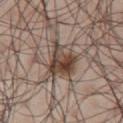Impression:
No biopsy was performed on this lesion — it was imaged during a full skin examination and was not determined to be concerning.
Context:
The lesion's longest dimension is about 5 mm. This is a white-light tile. A male subject, in their mid-40s. A close-up tile cropped from a whole-body skin photograph, about 15 mm across. From the right upper arm. The lesion-visualizer software estimated a shape eccentricity near 0.8 and a shape-asymmetry score of about 0.45 (0 = symmetric). It also reported roughly 14 lightness units darker than nearby skin and a lesion-to-skin contrast of about 10.5 (normalized; higher = more distinct). And it measured a border-irregularity index near 5.5/10, internal color variation of about 7.5 on a 0–10 scale, and radial color variation of about 2.5. And it measured lesion-presence confidence of about 95/100.